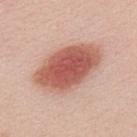• biopsy status — total-body-photography surveillance lesion; no biopsy
• acquisition — 15 mm crop, total-body photography
• site — the back
• size — ~8.5 mm (longest diameter)
• subject — male, approximately 25 years of age
• illumination — white-light